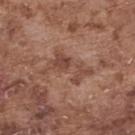The lesion was tiled from a total-body skin photograph and was not biopsied. The lesion is located on the upper back. Approximately 6 mm at its widest. The subject is a male aged 73 to 77. A region of skin cropped from a whole-body photographic capture, roughly 15 mm wide. The tile uses white-light illumination.